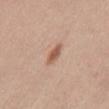No biopsy was performed on this lesion — it was imaged during a full skin examination and was not determined to be concerning. A 15 mm crop from a total-body photograph taken for skin-cancer surveillance. A male patient, aged 58 to 62. Located on the abdomen. Longest diameter approximately 3 mm. Imaged with white-light lighting.